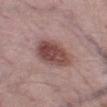Impression:
This lesion was catalogued during total-body skin photography and was not selected for biopsy.
Context:
The patient is a male aged around 50. The lesion is on the left thigh. Automated image analysis of the tile measured border irregularity of about 1.5 on a 0–10 scale, a within-lesion color-variation index near 5/10, and a peripheral color-asymmetry measure near 1.5. The analysis additionally found an automated nevus-likeness rating near 80 out of 100 and a lesion-detection confidence of about 100/100. Captured under white-light illumination. Longest diameter approximately 6 mm. A 15 mm close-up extracted from a 3D total-body photography capture.